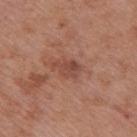workup = no biopsy performed (imaged during a skin exam) | subject = male, aged 68–72 | lesion size = about 3.5 mm | tile lighting = white-light | imaging modality = 15 mm crop, total-body photography | body site = the mid back.